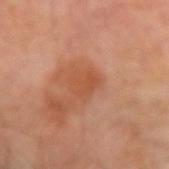  biopsy_status: not biopsied; imaged during a skin examination
  patient:
    sex: male
    age_approx: 70
  site: left forearm
  lighting: cross-polarized
  image:
    source: total-body photography crop
    field_of_view_mm: 15